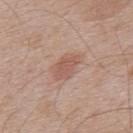biopsy status = no biopsy performed (imaged during a skin exam); subject = male, about 70 years old; anatomic site = the upper back; diameter = ≈4 mm; acquisition = total-body-photography crop, ~15 mm field of view.